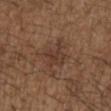Recorded during total-body skin imaging; not selected for excision or biopsy.
The subject is a male aged approximately 50.
A close-up tile cropped from a whole-body skin photograph, about 15 mm across.
The lesion is on the right upper arm.